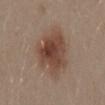This lesion was catalogued during total-body skin photography and was not selected for biopsy. Cropped from a total-body skin-imaging series; the visible field is about 15 mm. Imaged with white-light lighting. A male subject aged approximately 30. The lesion's longest dimension is about 6.5 mm. The lesion is on the mid back.